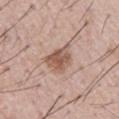Notes:
– biopsy status: no biopsy performed (imaged during a skin exam)
– illumination: white-light illumination
– acquisition: total-body-photography crop, ~15 mm field of view
– patient: male, aged around 55
– lesion diameter: ≈3.5 mm